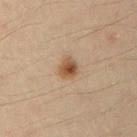| feature | finding |
|---|---|
| biopsy status | total-body-photography surveillance lesion; no biopsy |
| site | the arm |
| TBP lesion metrics | a border-irregularity index near 1.5/10, a within-lesion color-variation index near 5.5/10, and radial color variation of about 1.5; a classifier nevus-likeness of about 95/100 and a lesion-detection confidence of about 100/100 |
| subject | male, approximately 35 years of age |
| size | ~2.5 mm (longest diameter) |
| tile lighting | cross-polarized |
| image source | 15 mm crop, total-body photography |